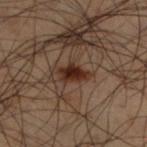Clinical impression: Imaged during a routine full-body skin examination; the lesion was not biopsied and no histopathology is available. Clinical summary: The lesion is located on the left lower leg. A 15 mm crop from a total-body photograph taken for skin-cancer surveillance. The recorded lesion diameter is about 4 mm. The subject is a male aged approximately 50. Imaged with cross-polarized lighting.